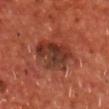No biopsy was performed on this lesion — it was imaged during a full skin examination and was not determined to be concerning.
A male subject aged approximately 70.
Automated tile analysis of the lesion measured a footprint of about 15 mm² and an eccentricity of roughly 0.65. The software also gave a mean CIELAB color near L≈34 a*≈26 b*≈28, about 10 CIELAB-L* units darker than the surrounding skin, and a lesion-to-skin contrast of about 8.5 (normalized; higher = more distinct).
A 15 mm close-up tile from a total-body photography series done for melanoma screening.
Captured under cross-polarized illumination.
On the chest.